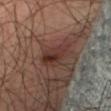The lesion is located on the right thigh. The total-body-photography lesion software estimated an eccentricity of roughly 0.8 and two-axis asymmetry of about 0.2. The analysis additionally found a mean CIELAB color near L≈33 a*≈19 b*≈22, roughly 8 lightness units darker than nearby skin, and a lesion-to-skin contrast of about 7.5 (normalized; higher = more distinct). And it measured border irregularity of about 2 on a 0–10 scale, a within-lesion color-variation index near 7.5/10, and a peripheral color-asymmetry measure near 3. Longest diameter approximately 4 mm. A 15 mm close-up tile from a total-body photography series done for melanoma screening. Captured under cross-polarized illumination. The subject is a male aged approximately 65.